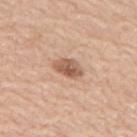Clinical impression: Imaged during a routine full-body skin examination; the lesion was not biopsied and no histopathology is available. Acquisition and patient details: Automated image analysis of the tile measured a border-irregularity rating of about 2.5/10, a color-variation rating of about 5/10, and radial color variation of about 1.5. The lesion is on the upper back. A region of skin cropped from a whole-body photographic capture, roughly 15 mm wide. This is a white-light tile. The patient is a male aged 68 to 72.